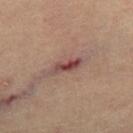Impression:
This lesion was catalogued during total-body skin photography and was not selected for biopsy.
Acquisition and patient details:
Longest diameter approximately 4.5 mm. Located on the left leg. A female subject approximately 65 years of age. The tile uses cross-polarized illumination. A roughly 15 mm field-of-view crop from a total-body skin photograph.